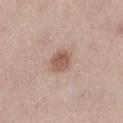Impression:
Captured during whole-body skin photography for melanoma surveillance; the lesion was not biopsied.
Image and clinical context:
A lesion tile, about 15 mm wide, cut from a 3D total-body photograph. Located on the right thigh. The subject is a female aged 38–42. Measured at roughly 3 mm in maximum diameter. The lesion-visualizer software estimated an outline eccentricity of about 0.6 (0 = round, 1 = elongated) and a shape-asymmetry score of about 0.15 (0 = symmetric). And it measured border irregularity of about 1.5 on a 0–10 scale. The tile uses white-light illumination.